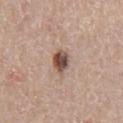Notes:
– image source: 15 mm crop, total-body photography
– tile lighting: white-light illumination
– location: the chest
– automated lesion analysis: a border-irregularity index near 2/10, internal color variation of about 7 on a 0–10 scale, and peripheral color asymmetry of about 2.5; an automated nevus-likeness rating near 95 out of 100 and lesion-presence confidence of about 100/100
– size: about 3 mm
– patient: male, about 65 years old